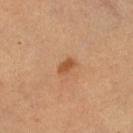{
  "biopsy_status": "not biopsied; imaged during a skin examination",
  "lesion_size": {
    "long_diameter_mm_approx": 2.0
  },
  "lighting": "cross-polarized",
  "image": {
    "source": "total-body photography crop",
    "field_of_view_mm": 15
  },
  "patient": {
    "sex": "female",
    "age_approx": 65
  },
  "site": "right lower leg",
  "automated_metrics": {
    "area_mm2_approx": 2.5,
    "eccentricity": 0.75,
    "shape_asymmetry": 0.25,
    "border_irregularity_0_10": 2.0,
    "color_variation_0_10": 1.0,
    "peripheral_color_asymmetry": 0.5
  }
}